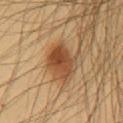Impression: The lesion was photographed on a routine skin check and not biopsied; there is no pathology result. Background: A male patient about 40 years old. On the chest. Cropped from a whole-body photographic skin survey; the tile spans about 15 mm. Automated image analysis of the tile measured a border-irregularity index near 2/10. The recorded lesion diameter is about 4 mm.